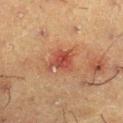Recorded during total-body skin imaging; not selected for excision or biopsy.
The lesion is located on the right lower leg.
Cropped from a whole-body photographic skin survey; the tile spans about 15 mm.
This is a cross-polarized tile.
The subject is a male aged around 60.
The recorded lesion diameter is about 3.5 mm.